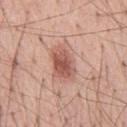<case>
  <biopsy_status>not biopsied; imaged during a skin examination</biopsy_status>
  <site>chest</site>
  <patient>
    <sex>male</sex>
    <age_approx>55</age_approx>
  </patient>
  <automated_metrics>
    <area_mm2_approx>9.5</area_mm2_approx>
    <eccentricity>0.8</eccentricity>
    <shape_asymmetry>0.25</shape_asymmetry>
    <cielab_L>56</cielab_L>
    <cielab_a>24</cielab_a>
    <cielab_b>27</cielab_b>
    <vs_skin_darker_L>12.0</vs_skin_darker_L>
    <vs_skin_contrast_norm>8.0</vs_skin_contrast_norm>
    <nevus_likeness_0_100>90</nevus_likeness_0_100>
    <lesion_detection_confidence_0_100>100</lesion_detection_confidence_0_100>
  </automated_metrics>
  <lighting>white-light</lighting>
  <image>
    <source>total-body photography crop</source>
    <field_of_view_mm>15</field_of_view_mm>
  </image>
</case>